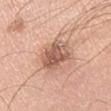{
  "biopsy_status": "not biopsied; imaged during a skin examination",
  "lesion_size": {
    "long_diameter_mm_approx": 4.5
  },
  "patient": {
    "sex": "male",
    "age_approx": 40
  },
  "lighting": "white-light",
  "image": {
    "source": "total-body photography crop",
    "field_of_view_mm": 15
  },
  "site": "arm"
}